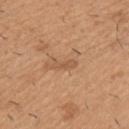{
  "biopsy_status": "not biopsied; imaged during a skin examination",
  "lighting": "white-light",
  "lesion_size": {
    "long_diameter_mm_approx": 3.0
  },
  "patient": {
    "sex": "male",
    "age_approx": 40
  },
  "site": "left upper arm",
  "automated_metrics": {
    "border_irregularity_0_10": 4.0,
    "color_variation_0_10": 0.0,
    "peripheral_color_asymmetry": 0.0,
    "nevus_likeness_0_100": 0,
    "lesion_detection_confidence_0_100": 95
  },
  "image": {
    "source": "total-body photography crop",
    "field_of_view_mm": 15
  }
}